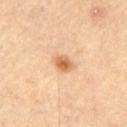  automated_metrics:
    area_mm2_approx: 4.0
    shape_asymmetry: 0.15
    vs_skin_darker_L: 13.0
    vs_skin_contrast_norm: 8.0
    border_irregularity_0_10: 1.5
    color_variation_0_10: 3.0
  patient:
    sex: male
    age_approx: 55
  site: left thigh
  image:
    source: total-body photography crop
    field_of_view_mm: 15
  lesion_size:
    long_diameter_mm_approx: 2.5